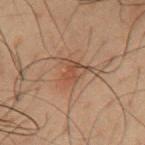No biopsy was performed on this lesion — it was imaged during a full skin examination and was not determined to be concerning.
On the mid back.
A lesion tile, about 15 mm wide, cut from a 3D total-body photograph.
Automated tile analysis of the lesion measured border irregularity of about 4.5 on a 0–10 scale, internal color variation of about 4 on a 0–10 scale, and a peripheral color-asymmetry measure near 1.5. The software also gave a nevus-likeness score of about 5/100 and a detector confidence of about 100 out of 100 that the crop contains a lesion.
A male subject, aged 48 to 52.
Approximately 3.5 mm at its widest.